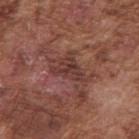<case>
<biopsy_status>not biopsied; imaged during a skin examination</biopsy_status>
<patient>
  <sex>male</sex>
  <age_approx>75</age_approx>
</patient>
<image>
  <source>total-body photography crop</source>
  <field_of_view_mm>15</field_of_view_mm>
</image>
<lesion_size>
  <long_diameter_mm_approx>5.0</long_diameter_mm_approx>
</lesion_size>
<lighting>white-light</lighting>
<automated_metrics>
  <area_mm2_approx>10.0</area_mm2_approx>
  <eccentricity>0.85</eccentricity>
  <shape_asymmetry>0.45</shape_asymmetry>
</automated_metrics>
<site>right upper arm</site>
</case>